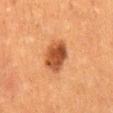  biopsy_status: not biopsied; imaged during a skin examination
  lesion_size:
    long_diameter_mm_approx: 4.0
  automated_metrics:
    vs_skin_darker_L: 13.0
    vs_skin_contrast_norm: 10.0
  site: mid back
  patient:
    sex: male
    age_approx: 50
  image:
    source: total-body photography crop
    field_of_view_mm: 15